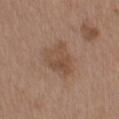Clinical impression: No biopsy was performed on this lesion — it was imaged during a full skin examination and was not determined to be concerning. Context: The subject is a male roughly 40 years of age. A 15 mm crop from a total-body photograph taken for skin-cancer surveillance. Approximately 4.5 mm at its widest. Captured under white-light illumination. The lesion is on the upper back.